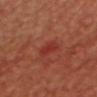<case>
  <biopsy_status>not biopsied; imaged during a skin examination</biopsy_status>
  <patient>
    <sex>female</sex>
    <age_approx>45</age_approx>
  </patient>
  <automated_metrics>
    <cielab_L>35</cielab_L>
    <cielab_a>34</cielab_a>
    <cielab_b>30</cielab_b>
    <vs_skin_darker_L>7.0</vs_skin_darker_L>
    <border_irregularity_0_10>3.0</border_irregularity_0_10>
    <color_variation_0_10>2.0</color_variation_0_10>
  </automated_metrics>
  <image>
    <source>total-body photography crop</source>
    <field_of_view_mm>15</field_of_view_mm>
  </image>
  <site>chest</site>
  <lighting>cross-polarized</lighting>
  <lesion_size>
    <long_diameter_mm_approx>2.5</long_diameter_mm_approx>
  </lesion_size>
</case>